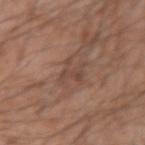This lesion was catalogued during total-body skin photography and was not selected for biopsy. A region of skin cropped from a whole-body photographic capture, roughly 15 mm wide. Automated tile analysis of the lesion measured a lesion area of about 5 mm² and a shape eccentricity near 0.75. The software also gave a mean CIELAB color near L≈45 a*≈18 b*≈25, a lesion–skin lightness drop of about 7, and a normalized lesion–skin contrast near 6. And it measured border irregularity of about 8.5 on a 0–10 scale and a peripheral color-asymmetry measure near 0.5. And it measured a nevus-likeness score of about 0/100 and a lesion-detection confidence of about 60/100. The patient is a male aged approximately 30. The tile uses white-light illumination. From the left forearm. The recorded lesion diameter is about 3.5 mm.